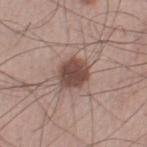Impression:
Part of a total-body skin-imaging series; this lesion was reviewed on a skin check and was not flagged for biopsy.
Acquisition and patient details:
A male subject, in their mid- to late 50s. Automated image analysis of the tile measured a lesion area of about 8.5 mm², a shape eccentricity near 0.5, and a symmetry-axis asymmetry near 0.15. It also reported a mean CIELAB color near L≈46 a*≈19 b*≈22 and a lesion-to-skin contrast of about 10.5 (normalized; higher = more distinct). It also reported a nevus-likeness score of about 85/100 and a lesion-detection confidence of about 100/100. Cropped from a whole-body photographic skin survey; the tile spans about 15 mm. About 3.5 mm across.